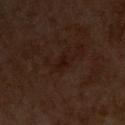Captured during whole-body skin photography for melanoma surveillance; the lesion was not biopsied. The patient is a male aged around 60. The lesion is located on the chest. The total-body-photography lesion software estimated an area of roughly 4 mm², an eccentricity of roughly 0.8, and a symmetry-axis asymmetry near 0.35. And it measured about 4 CIELAB-L* units darker than the surrounding skin. The lesion's longest dimension is about 3 mm. This is a cross-polarized tile. This image is a 15 mm lesion crop taken from a total-body photograph.